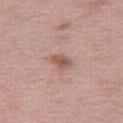Assessment:
Imaged during a routine full-body skin examination; the lesion was not biopsied and no histopathology is available.
Acquisition and patient details:
Located on the right thigh. The patient is a female approximately 40 years of age. A 15 mm close-up extracted from a 3D total-body photography capture.